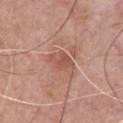– workup · total-body-photography surveillance lesion; no biopsy
– size · about 3 mm
– TBP lesion metrics · a classifier nevus-likeness of about 5/100 and lesion-presence confidence of about 100/100
– patient · male, aged approximately 60
– body site · the front of the torso
– illumination · white-light illumination
– acquisition · total-body-photography crop, ~15 mm field of view